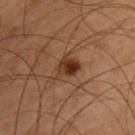Notes:
• notes — total-body-photography surveillance lesion; no biopsy
• diameter — ≈3 mm
• location — the upper back
• image — total-body-photography crop, ~15 mm field of view
• patient — male, in their mid-40s
• tile lighting — cross-polarized illumination